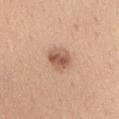workup = total-body-photography surveillance lesion; no biopsy
image source = 15 mm crop, total-body photography
anatomic site = the chest
subject = male, approximately 40 years of age